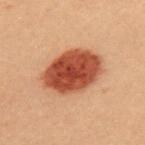Assessment: The lesion was photographed on a routine skin check and not biopsied; there is no pathology result.